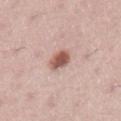  biopsy_status: not biopsied; imaged during a skin examination
  image:
    source: total-body photography crop
    field_of_view_mm: 15
  site: left lower leg
  patient:
    sex: female
    age_approx: 50
  lesion_size:
    long_diameter_mm_approx: 2.5
  automated_metrics:
    cielab_L: 56
    cielab_a: 22
    cielab_b: 26
    vs_skin_darker_L: 15.0
    vs_skin_contrast_norm: 10.0
    nevus_likeness_0_100: 95
    lesion_detection_confidence_0_100: 100